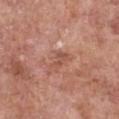follow-up — imaged on a skin check; not biopsied
image — ~15 mm crop, total-body skin-cancer survey
patient — female, approximately 75 years of age
anatomic site — the chest
size — ≈2.5 mm
lighting — white-light